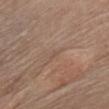The lesion was photographed on a routine skin check and not biopsied; there is no pathology result. A female patient aged 63–67. From the chest. A 15 mm close-up extracted from a 3D total-body photography capture.